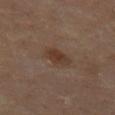Clinical impression:
Captured during whole-body skin photography for melanoma surveillance; the lesion was not biopsied.
Clinical summary:
This is a cross-polarized tile. A 15 mm close-up tile from a total-body photography series done for melanoma screening. The lesion's longest dimension is about 3.5 mm. Automated tile analysis of the lesion measured a lesion area of about 5.5 mm², an eccentricity of roughly 0.8, and a symmetry-axis asymmetry near 0.25. And it measured border irregularity of about 2.5 on a 0–10 scale, internal color variation of about 2.5 on a 0–10 scale, and a peripheral color-asymmetry measure near 1. A female patient aged around 50. Located on the left thigh.